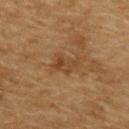Clinical impression:
Imaged during a routine full-body skin examination; the lesion was not biopsied and no histopathology is available.
Background:
A male subject, aged around 85 or older. Imaged with cross-polarized lighting. The recorded lesion diameter is about 3 mm. A region of skin cropped from a whole-body photographic capture, roughly 15 mm wide. Located on the back. The lesion-visualizer software estimated an area of roughly 4.5 mm² and an outline eccentricity of about 0.75 (0 = round, 1 = elongated). The analysis additionally found a lesion color around L≈37 a*≈17 b*≈31 in CIELAB, roughly 7 lightness units darker than nearby skin, and a lesion-to-skin contrast of about 6.5 (normalized; higher = more distinct). The analysis additionally found a classifier nevus-likeness of about 0/100.